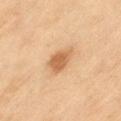Assessment: Imaged during a routine full-body skin examination; the lesion was not biopsied and no histopathology is available. Context: Longest diameter approximately 4 mm. The lesion is on the left thigh. A 15 mm crop from a total-body photograph taken for skin-cancer surveillance. The patient is a female about 60 years old. An algorithmic analysis of the crop reported a border-irregularity rating of about 2.5/10 and peripheral color asymmetry of about 1.